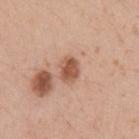| field | value |
|---|---|
| biopsy status | no biopsy performed (imaged during a skin exam) |
| anatomic site | the arm |
| subject | male, in their 30s |
| automated lesion analysis | an eccentricity of roughly 0.55 and a symmetry-axis asymmetry near 0.25; a border-irregularity rating of about 2/10 and peripheral color asymmetry of about 1; an automated nevus-likeness rating near 95 out of 100 and a detector confidence of about 100 out of 100 that the crop contains a lesion |
| imaging modality | total-body-photography crop, ~15 mm field of view |
| diameter | ~2.5 mm (longest diameter) |